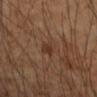Background:
This image is a 15 mm lesion crop taken from a total-body photograph. The subject is a female approximately 45 years of age. Captured under cross-polarized illumination. About 3 mm across. Located on the right upper arm.